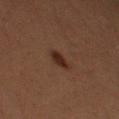notes — catalogued during a skin exam; not biopsied | lesion diameter — ≈2.5 mm | patient — female, aged 48–52 | TBP lesion metrics — a border-irregularity index near 2.5/10, a color-variation rating of about 1.5/10, and radial color variation of about 0.5 | acquisition — ~15 mm crop, total-body skin-cancer survey | site — the left thigh.